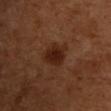Context: About 3.5 mm across. A roughly 15 mm field-of-view crop from a total-body skin photograph. The lesion is on the chest. A male patient, about 60 years old. Captured under cross-polarized illumination.